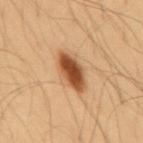<tbp_lesion>
  <biopsy_status>not biopsied; imaged during a skin examination</biopsy_status>
  <patient>
    <sex>male</sex>
    <age_approx>55</age_approx>
  </patient>
  <site>mid back</site>
  <image>
    <source>total-body photography crop</source>
    <field_of_view_mm>15</field_of_view_mm>
  </image>
</tbp_lesion>